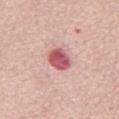Captured during whole-body skin photography for melanoma surveillance; the lesion was not biopsied.
About 3.5 mm across.
Automated image analysis of the tile measured an area of roughly 7 mm² and an eccentricity of roughly 0.6. It also reported a mean CIELAB color near L≈57 a*≈32 b*≈21, roughly 17 lightness units darker than nearby skin, and a lesion-to-skin contrast of about 10.5 (normalized; higher = more distinct). It also reported a border-irregularity index near 1.5/10, a within-lesion color-variation index near 5/10, and a peripheral color-asymmetry measure near 1.5. And it measured a classifier nevus-likeness of about 0/100 and a lesion-detection confidence of about 100/100.
A 15 mm crop from a total-body photograph taken for skin-cancer surveillance.
A male patient, aged approximately 55.
The tile uses white-light illumination.